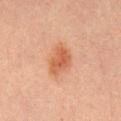biopsy_status: not biopsied; imaged during a skin examination
site: mid back
image:
  source: total-body photography crop
  field_of_view_mm: 15
patient:
  sex: male
  age_approx: 30